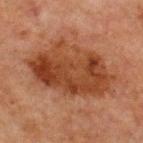This lesion was catalogued during total-body skin photography and was not selected for biopsy.
A male subject, in their mid- to late 60s.
Imaged with cross-polarized lighting.
From the front of the torso.
A 15 mm close-up extracted from a 3D total-body photography capture.